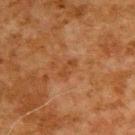Impression:
Part of a total-body skin-imaging series; this lesion was reviewed on a skin check and was not flagged for biopsy.